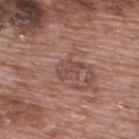Assessment: The lesion was photographed on a routine skin check and not biopsied; there is no pathology result.